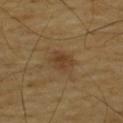Assessment: Part of a total-body skin-imaging series; this lesion was reviewed on a skin check and was not flagged for biopsy. Background: The total-body-photography lesion software estimated a lesion color around L≈40 a*≈16 b*≈33 in CIELAB and about 7 CIELAB-L* units darker than the surrounding skin. The tile uses cross-polarized illumination. A close-up tile cropped from a whole-body skin photograph, about 15 mm across. The lesion's longest dimension is about 2.5 mm. The lesion is located on the upper back. A male subject, aged 63 to 67.